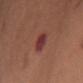{
  "biopsy_status": "not biopsied; imaged during a skin examination",
  "image": {
    "source": "total-body photography crop",
    "field_of_view_mm": 15
  },
  "patient": {
    "sex": "female",
    "age_approx": 65
  },
  "site": "chest",
  "lighting": "white-light",
  "lesion_size": {
    "long_diameter_mm_approx": 3.0
  }
}